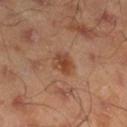Acquisition and patient details: The lesion's longest dimension is about 2.5 mm. On the right lower leg. Cropped from a total-body skin-imaging series; the visible field is about 15 mm. The patient is a male aged 43–47.